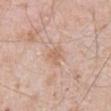No biopsy was performed on this lesion — it was imaged during a full skin examination and was not determined to be concerning. Cropped from a whole-body photographic skin survey; the tile spans about 15 mm. Automated image analysis of the tile measured border irregularity of about 3.5 on a 0–10 scale, internal color variation of about 1.5 on a 0–10 scale, and radial color variation of about 0.5. The software also gave an automated nevus-likeness rating near 0 out of 100 and a lesion-detection confidence of about 100/100. The patient is a male aged around 80. The lesion is on the front of the torso.